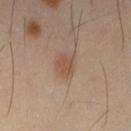biopsy_status: not biopsied; imaged during a skin examination
lighting: cross-polarized
patient:
  sex: male
  age_approx: 40
site: right upper arm
image:
  source: total-body photography crop
  field_of_view_mm: 15